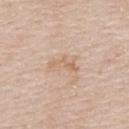A female patient approximately 55 years of age. This is a white-light tile. The total-body-photography lesion software estimated an area of roughly 3.5 mm², a shape eccentricity near 0.95, and a shape-asymmetry score of about 0.5 (0 = symmetric). The software also gave internal color variation of about 0 on a 0–10 scale. The analysis additionally found a nevus-likeness score of about 0/100 and a lesion-detection confidence of about 100/100. From the upper back. About 3.5 mm across. A lesion tile, about 15 mm wide, cut from a 3D total-body photograph.